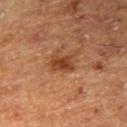Clinical impression:
The lesion was photographed on a routine skin check and not biopsied; there is no pathology result.
Context:
Captured under cross-polarized illumination. Approximately 3 mm at its widest. The subject is a male aged around 75. From the right thigh. A 15 mm close-up extracted from a 3D total-body photography capture.